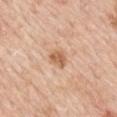Impression: The lesion was photographed on a routine skin check and not biopsied; there is no pathology result. Image and clinical context: Automated image analysis of the tile measured a lesion area of about 4.5 mm² and a symmetry-axis asymmetry near 0.2. It also reported a nevus-likeness score of about 50/100. A male subject, aged approximately 60. Captured under white-light illumination. From the back. A 15 mm crop from a total-body photograph taken for skin-cancer surveillance. Measured at roughly 2.5 mm in maximum diameter.